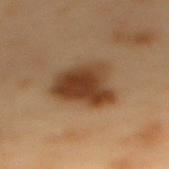follow-up: catalogued during a skin exam; not biopsied | imaging modality: ~15 mm tile from a whole-body skin photo | illumination: cross-polarized illumination | lesion diameter: about 5.5 mm | location: the mid back | patient: male, in their mid- to late 50s.